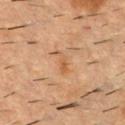Q: Was this lesion biopsied?
A: no biopsy performed (imaged during a skin exam)
Q: Who is the patient?
A: male, in their mid- to late 50s
Q: Automated lesion metrics?
A: an eccentricity of roughly 0.9 and two-axis asymmetry of about 0.4; a border-irregularity index near 4.5/10, internal color variation of about 0 on a 0–10 scale, and radial color variation of about 0
Q: What is the imaging modality?
A: total-body-photography crop, ~15 mm field of view
Q: How was the tile lit?
A: cross-polarized illumination
Q: What is the anatomic site?
A: the upper back